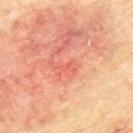{
  "biopsy_status": "not biopsied; imaged during a skin examination",
  "patient": {
    "sex": "male",
    "age_approx": 70
  },
  "site": "upper back",
  "image": {
    "source": "total-body photography crop",
    "field_of_view_mm": 15
  }
}